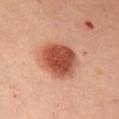workup: catalogued during a skin exam; not biopsied
patient: female, about 45 years old
lighting: cross-polarized
image-analysis metrics: lesion-presence confidence of about 100/100
site: the right upper arm
lesion size: about 5 mm
image source: ~15 mm tile from a whole-body skin photo